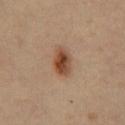Captured during whole-body skin photography for melanoma surveillance; the lesion was not biopsied.
The lesion is located on the chest.
Automated tile analysis of the lesion measured about 12 CIELAB-L* units darker than the surrounding skin and a lesion-to-skin contrast of about 10 (normalized; higher = more distinct). And it measured border irregularity of about 2 on a 0–10 scale, a within-lesion color-variation index near 6.5/10, and a peripheral color-asymmetry measure near 2.
A region of skin cropped from a whole-body photographic capture, roughly 15 mm wide.
A male patient, aged around 55.
Approximately 4 mm at its widest.
Imaged with cross-polarized lighting.